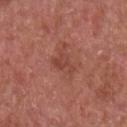Captured during whole-body skin photography for melanoma surveillance; the lesion was not biopsied.
The tile uses white-light illumination.
A male patient about 65 years old.
A 15 mm close-up tile from a total-body photography series done for melanoma screening.
On the right upper arm.
The lesion's longest dimension is about 3 mm.